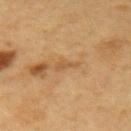biopsy status: no biopsy performed (imaged during a skin exam); lighting: cross-polarized illumination; size: ~2.5 mm (longest diameter); patient: female, aged 43–47; image-analysis metrics: a border-irregularity rating of about 5/10, a within-lesion color-variation index near 0.5/10, and a peripheral color-asymmetry measure near 0; image source: total-body-photography crop, ~15 mm field of view; body site: the right forearm.